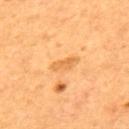Assessment:
Recorded during total-body skin imaging; not selected for excision or biopsy.
Acquisition and patient details:
Cropped from a total-body skin-imaging series; the visible field is about 15 mm. The lesion's longest dimension is about 3 mm. This is a cross-polarized tile. The subject is a male aged approximately 55. The total-body-photography lesion software estimated a lesion color around L≈62 a*≈24 b*≈46 in CIELAB and a lesion–skin lightness drop of about 8. The analysis additionally found a classifier nevus-likeness of about 0/100. Located on the upper back.